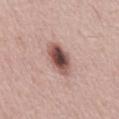<record>
<biopsy_status>not biopsied; imaged during a skin examination</biopsy_status>
<site>mid back</site>
<automated_metrics>
  <eccentricity>0.8</eccentricity>
  <shape_asymmetry>0.2</shape_asymmetry>
</automated_metrics>
<lesion_size>
  <long_diameter_mm_approx>4.5</long_diameter_mm_approx>
</lesion_size>
<image>
  <source>total-body photography crop</source>
  <field_of_view_mm>15</field_of_view_mm>
</image>
<patient>
  <sex>male</sex>
  <age_approx>65</age_approx>
</patient>
</record>